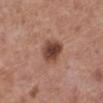Imaged during a routine full-body skin examination; the lesion was not biopsied and no histopathology is available.
Located on the leg.
About 3.5 mm across.
Imaged with white-light lighting.
The patient is a female aged approximately 50.
Cropped from a total-body skin-imaging series; the visible field is about 15 mm.